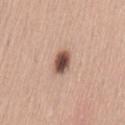Impression: Part of a total-body skin-imaging series; this lesion was reviewed on a skin check and was not flagged for biopsy. Context: The lesion is on the lower back. A region of skin cropped from a whole-body photographic capture, roughly 15 mm wide. This is a white-light tile. Measured at roughly 3 mm in maximum diameter. A female subject, about 30 years old.